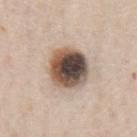Case summary:
- follow-up: no biopsy performed (imaged during a skin exam)
- lighting: white-light
- location: the chest
- automated lesion analysis: a lesion color around L≈50 a*≈14 b*≈24 in CIELAB, a lesion–skin lightness drop of about 22, and a normalized lesion–skin contrast near 14.5; a color-variation rating of about 9.5/10 and peripheral color asymmetry of about 3
- image: 15 mm crop, total-body photography
- lesion diameter: ≈5 mm
- patient: male, aged around 60